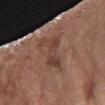This lesion was catalogued during total-body skin photography and was not selected for biopsy.
Automated image analysis of the tile measured an area of roughly 11 mm², an outline eccentricity of about 0.95 (0 = round, 1 = elongated), and a symmetry-axis asymmetry near 0.45. It also reported an average lesion color of about L≈42 a*≈19 b*≈25 (CIELAB) and a lesion-to-skin contrast of about 6 (normalized; higher = more distinct). And it measured a border-irregularity index near 7/10. The software also gave a nevus-likeness score of about 0/100 and a lesion-detection confidence of about 65/100.
The subject is a female aged 73 to 77.
The lesion is located on the right forearm.
About 7 mm across.
A region of skin cropped from a whole-body photographic capture, roughly 15 mm wide.